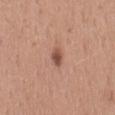Recorded during total-body skin imaging; not selected for excision or biopsy. About 2.5 mm across. A close-up tile cropped from a whole-body skin photograph, about 15 mm across. A female subject, roughly 35 years of age. This is a white-light tile. On the abdomen.